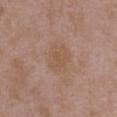follow-up: catalogued during a skin exam; not biopsied
TBP lesion metrics: about 5 CIELAB-L* units darker than the surrounding skin; a border-irregularity index near 2/10, internal color variation of about 2 on a 0–10 scale, and radial color variation of about 0.5
subject: female, in their mid-30s
diameter: ≈3.5 mm
site: the chest
acquisition: ~15 mm tile from a whole-body skin photo